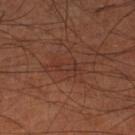notes = no biopsy performed (imaged during a skin exam)
site = the left lower leg
patient = male, about 70 years old
lighting = cross-polarized
image source = ~15 mm crop, total-body skin-cancer survey
lesion diameter = ~3.5 mm (longest diameter)